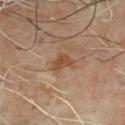<case>
<biopsy_status>not biopsied; imaged during a skin examination</biopsy_status>
<lighting>cross-polarized</lighting>
<site>chest</site>
<image>
  <source>total-body photography crop</source>
  <field_of_view_mm>15</field_of_view_mm>
</image>
<patient>
  <sex>male</sex>
  <age_approx>65</age_approx>
</patient>
<automated_metrics>
  <cielab_L>46</cielab_L>
  <cielab_a>19</cielab_a>
  <cielab_b>31</cielab_b>
  <vs_skin_darker_L>8.0</vs_skin_darker_L>
  <vs_skin_contrast_norm>7.5</vs_skin_contrast_norm>
</automated_metrics>
<lesion_size>
  <long_diameter_mm_approx>3.0</long_diameter_mm_approx>
</lesion_size>
</case>